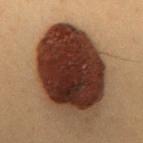biopsy status — no biopsy performed (imaged during a skin exam); subject — female, aged 38–42; size — ~11 mm (longest diameter); body site — the mid back; acquisition — 15 mm crop, total-body photography; lighting — cross-polarized illumination.